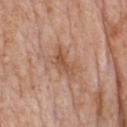The lesion was photographed on a routine skin check and not biopsied; there is no pathology result. Automated tile analysis of the lesion measured a lesion area of about 5.5 mm², a shape eccentricity near 0.9, and two-axis asymmetry of about 0.45. It also reported roughly 9 lightness units darker than nearby skin and a normalized lesion–skin contrast near 6.5. The software also gave an automated nevus-likeness rating near 0 out of 100 and a lesion-detection confidence of about 100/100. A 15 mm crop from a total-body photograph taken for skin-cancer surveillance. The recorded lesion diameter is about 4 mm. Imaged with white-light lighting. The lesion is located on the chest. A female patient, about 70 years old.